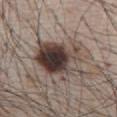Impression:
This lesion was catalogued during total-body skin photography and was not selected for biopsy.
Background:
A male subject aged around 65. An algorithmic analysis of the crop reported a footprint of about 23 mm² and an outline eccentricity of about 0.6 (0 = round, 1 = elongated). The analysis additionally found a lesion color around L≈40 a*≈13 b*≈19 in CIELAB, about 16 CIELAB-L* units darker than the surrounding skin, and a normalized border contrast of about 12.5. And it measured a border-irregularity rating of about 4/10, a color-variation rating of about 10/10, and peripheral color asymmetry of about 4.5. And it measured a nevus-likeness score of about 90/100 and a lesion-detection confidence of about 100/100. Captured under white-light illumination. The lesion is located on the chest. A 15 mm close-up extracted from a 3D total-body photography capture. Approximately 6 mm at its widest.